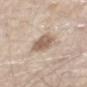This lesion was catalogued during total-body skin photography and was not selected for biopsy.